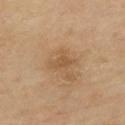biopsy status: no biopsy performed (imaged during a skin exam) | image: ~15 mm tile from a whole-body skin photo | site: the upper back | subject: female, aged 43 to 47 | tile lighting: cross-polarized | automated lesion analysis: a footprint of about 3.5 mm² and an outline eccentricity of about 0.8 (0 = round, 1 = elongated); roughly 7 lightness units darker than nearby skin; a border-irregularity index near 3/10, a color-variation rating of about 1/10, and peripheral color asymmetry of about 0.5.